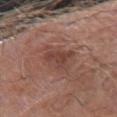Recorded during total-body skin imaging; not selected for excision or biopsy.
An algorithmic analysis of the crop reported a shape eccentricity near 0.9 and two-axis asymmetry of about 0.25. The analysis additionally found a border-irregularity rating of about 3/10, a within-lesion color-variation index near 4/10, and a peripheral color-asymmetry measure near 1.5. And it measured a classifier nevus-likeness of about 0/100 and a detector confidence of about 100 out of 100 that the crop contains a lesion.
The lesion's longest dimension is about 5.5 mm.
From the right forearm.
A male subject roughly 65 years of age.
A close-up tile cropped from a whole-body skin photograph, about 15 mm across.
Imaged with white-light lighting.